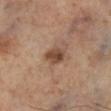Recorded during total-body skin imaging; not selected for excision or biopsy. On the right lower leg. The lesion-visualizer software estimated a lesion area of about 4.5 mm² and an outline eccentricity of about 0.7 (0 = round, 1 = elongated). The analysis additionally found a border-irregularity index near 2/10, internal color variation of about 3.5 on a 0–10 scale, and peripheral color asymmetry of about 1. The analysis additionally found an automated nevus-likeness rating near 80 out of 100 and lesion-presence confidence of about 100/100. Approximately 2.5 mm at its widest. A 15 mm close-up extracted from a 3D total-body photography capture.